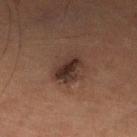Captured during whole-body skin photography for melanoma surveillance; the lesion was not biopsied.